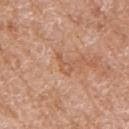Impression:
The lesion was photographed on a routine skin check and not biopsied; there is no pathology result.
Image and clinical context:
Located on the right upper arm. The patient is a female about 75 years old. Cropped from a total-body skin-imaging series; the visible field is about 15 mm.